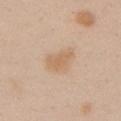<case>
  <biopsy_status>not biopsied; imaged during a skin examination</biopsy_status>
  <lighting>white-light</lighting>
  <site>left upper arm</site>
  <patient>
    <sex>female</sex>
    <age_approx>25</age_approx>
  </patient>
  <automated_metrics>
    <cielab_L>64</cielab_L>
    <cielab_a>17</cielab_a>
    <cielab_b>34</cielab_b>
    <vs_skin_darker_L>7.0</vs_skin_darker_L>
    <vs_skin_contrast_norm>6.0</vs_skin_contrast_norm>
    <lesion_detection_confidence_0_100>100</lesion_detection_confidence_0_100>
  </automated_metrics>
  <image>
    <source>total-body photography crop</source>
    <field_of_view_mm>15</field_of_view_mm>
  </image>
  <lesion_size>
    <long_diameter_mm_approx>4.0</long_diameter_mm_approx>
  </lesion_size>
</case>